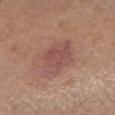notes: no biopsy performed (imaged during a skin exam)
lesion diameter: ≈5 mm
body site: the right forearm
automated metrics: a lesion color around L≈50 a*≈23 b*≈22 in CIELAB, about 8 CIELAB-L* units darker than the surrounding skin, and a normalized border contrast of about 7; a border-irregularity rating of about 2.5/10, a color-variation rating of about 3/10, and a peripheral color-asymmetry measure near 1; an automated nevus-likeness rating near 5 out of 100 and lesion-presence confidence of about 100/100
illumination: white-light illumination
patient: male, roughly 65 years of age
image source: ~15 mm tile from a whole-body skin photo